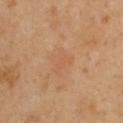<lesion>
  <biopsy_status>not biopsied; imaged during a skin examination</biopsy_status>
  <patient>
    <sex>female</sex>
    <age_approx>35</age_approx>
  </patient>
  <site>upper back</site>
  <lesion_size>
    <long_diameter_mm_approx>3.0</long_diameter_mm_approx>
  </lesion_size>
  <image>
    <source>total-body photography crop</source>
    <field_of_view_mm>15</field_of_view_mm>
  </image>
  <lighting>cross-polarized</lighting>
</lesion>